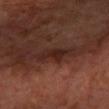{"biopsy_status": "not biopsied; imaged during a skin examination", "lighting": "cross-polarized", "image": {"source": "total-body photography crop", "field_of_view_mm": 15}, "patient": {"sex": "female", "age_approx": 65}, "lesion_size": {"long_diameter_mm_approx": 3.5}, "site": "left forearm"}